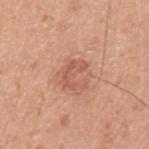patient: male, aged around 45; image: ~15 mm crop, total-body skin-cancer survey; illumination: white-light illumination; body site: the left upper arm.